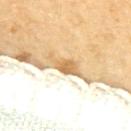follow-up: imaged on a skin check; not biopsied | anatomic site: the back | subject: female, aged approximately 70 | imaging modality: ~15 mm tile from a whole-body skin photo | lesion diameter: ≈4.5 mm | image-analysis metrics: a lesion color around L≈72 a*≈17 b*≈43 in CIELAB and roughly 15 lightness units darker than nearby skin; internal color variation of about 2.5 on a 0–10 scale and peripheral color asymmetry of about 0.5; a classifier nevus-likeness of about 0/100.